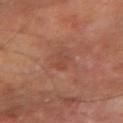Impression: The lesion was photographed on a routine skin check and not biopsied; there is no pathology result. Clinical summary: A male subject, roughly 60 years of age. Captured under cross-polarized illumination. Located on the left forearm. This image is a 15 mm lesion crop taken from a total-body photograph.